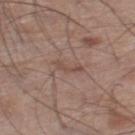Q: Was a biopsy performed?
A: total-body-photography surveillance lesion; no biopsy
Q: Patient demographics?
A: male, aged 68 to 72
Q: What lighting was used for the tile?
A: white-light illumination
Q: What is the imaging modality?
A: 15 mm crop, total-body photography
Q: What is the lesion's diameter?
A: ~3 mm (longest diameter)
Q: What is the anatomic site?
A: the left thigh